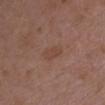Recorded during total-body skin imaging; not selected for excision or biopsy.
Longest diameter approximately 3.5 mm.
A lesion tile, about 15 mm wide, cut from a 3D total-body photograph.
From the chest.
A female subject roughly 35 years of age.